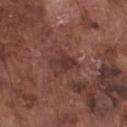diameter = about 3.5 mm
automated metrics = a lesion color around L≈35 a*≈21 b*≈22 in CIELAB, about 8 CIELAB-L* units darker than the surrounding skin, and a normalized border contrast of about 7; a detector confidence of about 95 out of 100 that the crop contains a lesion
image source = total-body-photography crop, ~15 mm field of view
patient = male, roughly 75 years of age
anatomic site = the front of the torso
illumination = white-light illumination